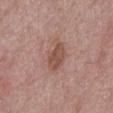{
  "biopsy_status": "not biopsied; imaged during a skin examination",
  "lesion_size": {
    "long_diameter_mm_approx": 4.5
  },
  "lighting": "white-light",
  "patient": {
    "sex": "male",
    "age_approx": 65
  },
  "image": {
    "source": "total-body photography crop",
    "field_of_view_mm": 15
  },
  "site": "chest",
  "automated_metrics": {
    "border_irregularity_0_10": 3.0,
    "peripheral_color_asymmetry": 1.0
  }
}